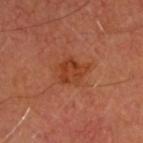Image and clinical context: A 15 mm close-up extracted from a 3D total-body photography capture. The subject is aged 63 to 67. The tile uses cross-polarized illumination. On the head or neck.